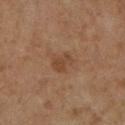Captured during whole-body skin photography for melanoma surveillance; the lesion was not biopsied. A roughly 15 mm field-of-view crop from a total-body skin photograph. The patient is a female in their mid- to late 60s. The lesion is located on the right lower leg. The recorded lesion diameter is about 3 mm.